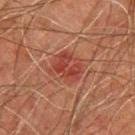This lesion was catalogued during total-body skin photography and was not selected for biopsy. From the upper back. The lesion's longest dimension is about 3.5 mm. This image is a 15 mm lesion crop taken from a total-body photograph. The tile uses cross-polarized illumination. Automated tile analysis of the lesion measured border irregularity of about 3 on a 0–10 scale and a peripheral color-asymmetry measure near 2. A male subject, aged approximately 45.